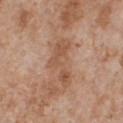{
  "biopsy_status": "not biopsied; imaged during a skin examination",
  "lesion_size": {
    "long_diameter_mm_approx": 6.0
  },
  "patient": {
    "sex": "male",
    "age_approx": 65
  },
  "site": "chest",
  "image": {
    "source": "total-body photography crop",
    "field_of_view_mm": 15
  },
  "lighting": "white-light"
}